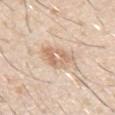Clinical impression: The lesion was tiled from a total-body skin photograph and was not biopsied.